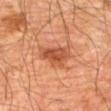Part of a total-body skin-imaging series; this lesion was reviewed on a skin check and was not flagged for biopsy. The tile uses cross-polarized illumination. The lesion is on the abdomen. Approximately 4.5 mm at its widest. A 15 mm close-up extracted from a 3D total-body photography capture. The patient is a male roughly 80 years of age.